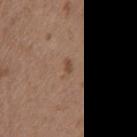Located on the chest. A female subject aged 63 to 67. A 15 mm close-up extracted from a 3D total-body photography capture.